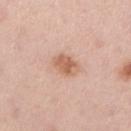Clinical impression:
Part of a total-body skin-imaging series; this lesion was reviewed on a skin check and was not flagged for biopsy.
Context:
The lesion-visualizer software estimated a lesion area of about 6 mm² and an eccentricity of roughly 0.75. And it measured a mean CIELAB color near L≈62 a*≈22 b*≈32, roughly 11 lightness units darker than nearby skin, and a normalized lesion–skin contrast near 7.5. On the leg. A female subject, aged approximately 45. Measured at roughly 3 mm in maximum diameter. This is a white-light tile. Cropped from a total-body skin-imaging series; the visible field is about 15 mm.